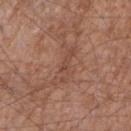The lesion was tiled from a total-body skin photograph and was not biopsied. A male patient, aged approximately 55. The lesion is located on the right forearm. A 15 mm close-up tile from a total-body photography series done for melanoma screening.